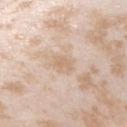Clinical impression: Captured during whole-body skin photography for melanoma surveillance; the lesion was not biopsied. Image and clinical context: The lesion is on the arm. A region of skin cropped from a whole-body photographic capture, roughly 15 mm wide. The recorded lesion diameter is about 2.5 mm. A female subject, aged 23–27.